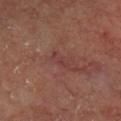Clinical impression: No biopsy was performed on this lesion — it was imaged during a full skin examination and was not determined to be concerning. Clinical summary: This image is a 15 mm lesion crop taken from a total-body photograph. The tile uses cross-polarized illumination. Longest diameter approximately 2.5 mm. The subject is a male aged 63 to 67. The lesion is on the left lower leg.